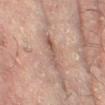Q: Was this lesion biopsied?
A: imaged on a skin check; not biopsied
Q: Automated lesion metrics?
A: a footprint of about 6.5 mm², a shape eccentricity near 0.9, and two-axis asymmetry of about 0.3; a border-irregularity rating of about 4/10, a color-variation rating of about 5.5/10, and radial color variation of about 2
Q: Where on the body is the lesion?
A: the left leg
Q: What kind of image is this?
A: total-body-photography crop, ~15 mm field of view
Q: How was the tile lit?
A: cross-polarized illumination
Q: Patient demographics?
A: female, approximately 80 years of age